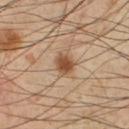Notes:
– notes: catalogued during a skin exam; not biopsied
– image: 15 mm crop, total-body photography
– lesion diameter: about 3 mm
– site: the left lower leg
– patient: male, in their mid-50s